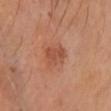Clinical summary:
The total-body-photography lesion software estimated an eccentricity of roughly 0.8. It also reported a mean CIELAB color near L≈51 a*≈27 b*≈33, roughly 8 lightness units darker than nearby skin, and a lesion-to-skin contrast of about 6 (normalized; higher = more distinct). A female subject, aged around 60. On the head or neck. A region of skin cropped from a whole-body photographic capture, roughly 15 mm wide. Captured under cross-polarized illumination. Approximately 3.5 mm at its widest.